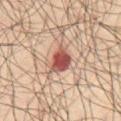tile lighting = cross-polarized
image = total-body-photography crop, ~15 mm field of view
diameter = ~3.5 mm (longest diameter)
automated metrics = a border-irregularity index near 2.5/10, a within-lesion color-variation index near 4.5/10, and a peripheral color-asymmetry measure near 1.5; a classifier nevus-likeness of about 15/100
subject = male, in their mid-60s
body site = the abdomen